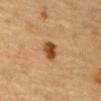Assessment:
The lesion was tiled from a total-body skin photograph and was not biopsied.
Clinical summary:
The lesion is on the abdomen. The tile uses cross-polarized illumination. The lesion-visualizer software estimated a footprint of about 5 mm² and a symmetry-axis asymmetry near 0.2. The analysis additionally found a mean CIELAB color near L≈41 a*≈19 b*≈35 and roughly 13 lightness units darker than nearby skin. The software also gave an automated nevus-likeness rating near 95 out of 100 and a detector confidence of about 100 out of 100 that the crop contains a lesion. Longest diameter approximately 2.5 mm. A male patient, roughly 85 years of age or older. A 15 mm close-up tile from a total-body photography series done for melanoma screening.